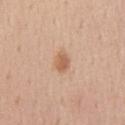This lesion was catalogued during total-body skin photography and was not selected for biopsy.
The lesion is located on the chest.
A lesion tile, about 15 mm wide, cut from a 3D total-body photograph.
The tile uses white-light illumination.
The recorded lesion diameter is about 3 mm.
A male patient roughly 55 years of age.